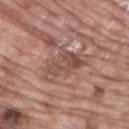Q: Is there a histopathology result?
A: imaged on a skin check; not biopsied
Q: Lesion size?
A: ≈5.5 mm
Q: How was the tile lit?
A: white-light
Q: Lesion location?
A: the upper back
Q: How was this image acquired?
A: total-body-photography crop, ~15 mm field of view
Q: Who is the patient?
A: male, aged approximately 70